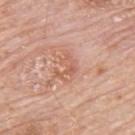A male patient in their 80s. A 15 mm close-up tile from a total-body photography series done for melanoma screening. The tile uses white-light illumination. The lesion's longest dimension is about 3.5 mm. On the back.